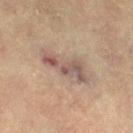{"biopsy_status": "not biopsied; imaged during a skin examination", "lighting": "cross-polarized", "patient": {"sex": "female", "age_approx": 80}, "image": {"source": "total-body photography crop", "field_of_view_mm": 15}, "lesion_size": {"long_diameter_mm_approx": 6.5}, "site": "leg"}